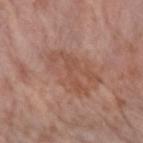  biopsy_status: not biopsied; imaged during a skin examination
  lighting: white-light
  patient:
    sex: female
    age_approx: 85+
  image:
    source: total-body photography crop
    field_of_view_mm: 15
  site: left forearm
  lesion_size:
    long_diameter_mm_approx: 5.5